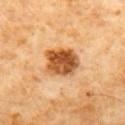Findings:
* biopsy status · no biopsy performed (imaged during a skin exam)
* imaging modality · total-body-photography crop, ~15 mm field of view
* illumination · cross-polarized illumination
* subject · male, aged 58 to 62
* size · ~4 mm (longest diameter)
* anatomic site · the front of the torso